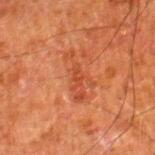Q: What kind of image is this?
A: 15 mm crop, total-body photography
Q: How was the tile lit?
A: cross-polarized
Q: Patient demographics?
A: male, approximately 80 years of age
Q: Lesion size?
A: ≈2.5 mm
Q: Where on the body is the lesion?
A: the right lower leg